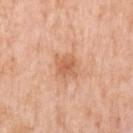Recorded during total-body skin imaging; not selected for excision or biopsy.